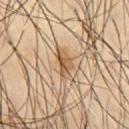workup = imaged on a skin check; not biopsied
location = the chest
lighting = cross-polarized illumination
subject = male, aged 53 to 57
size = ~3.5 mm (longest diameter)
image source = total-body-photography crop, ~15 mm field of view